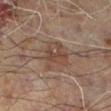{"biopsy_status": "not biopsied; imaged during a skin examination", "image": {"source": "total-body photography crop", "field_of_view_mm": 15}, "site": "leg", "patient": {"sex": "male", "age_approx": 60}, "lesion_size": {"long_diameter_mm_approx": 4.0}, "lighting": "cross-polarized"}